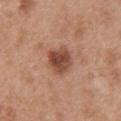workup — imaged on a skin check; not biopsied
anatomic site — the back
image source — total-body-photography crop, ~15 mm field of view
patient — male, in their 50s